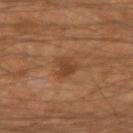Impression: Part of a total-body skin-imaging series; this lesion was reviewed on a skin check and was not flagged for biopsy. Image and clinical context: This is a cross-polarized tile. Approximately 3 mm at its widest. A 15 mm close-up extracted from a 3D total-body photography capture. The subject is a male in their mid- to late 40s. Located on the right upper arm. Automated image analysis of the tile measured a mean CIELAB color near L≈41 a*≈22 b*≈32 and a normalized lesion–skin contrast near 6.5. The analysis additionally found radial color variation of about 0.5.